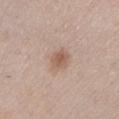Clinical impression:
The lesion was photographed on a routine skin check and not biopsied; there is no pathology result.
Acquisition and patient details:
Cropped from a total-body skin-imaging series; the visible field is about 15 mm. Located on the left lower leg. Automated image analysis of the tile measured a lesion area of about 5.5 mm², a shape eccentricity near 0.4, and a shape-asymmetry score of about 0.2 (0 = symmetric). The software also gave an average lesion color of about L≈58 a*≈18 b*≈28 (CIELAB), about 9 CIELAB-L* units darker than the surrounding skin, and a normalized border contrast of about 6.5. A female subject, aged around 40.